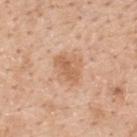Notes:
* notes · total-body-photography surveillance lesion; no biopsy
* size · ~3.5 mm (longest diameter)
* site · the upper back
* subject · female, approximately 55 years of age
* image · total-body-photography crop, ~15 mm field of view
* tile lighting · white-light illumination